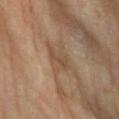Assessment:
Recorded during total-body skin imaging; not selected for excision or biopsy.
Background:
The subject is a female aged 68–72. A close-up tile cropped from a whole-body skin photograph, about 15 mm across. This is a cross-polarized tile. The lesion is on the right lower leg. The lesion-visualizer software estimated a footprint of about 3.5 mm², an outline eccentricity of about 0.95 (0 = round, 1 = elongated), and a shape-asymmetry score of about 0.4 (0 = symmetric). It also reported an average lesion color of about L≈49 a*≈17 b*≈31 (CIELAB), roughly 7 lightness units darker than nearby skin, and a normalized lesion–skin contrast near 6. And it measured a border-irregularity rating of about 5/10 and radial color variation of about 0. Measured at roughly 3.5 mm in maximum diameter.